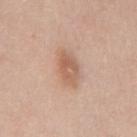Findings:
- workup — catalogued during a skin exam; not biopsied
- patient — male, roughly 60 years of age
- image source — 15 mm crop, total-body photography
- automated metrics — a lesion area of about 8 mm², a shape eccentricity near 0.8, and two-axis asymmetry of about 0.2; a lesion-detection confidence of about 100/100
- site — the mid back
- tile lighting — white-light